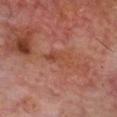patient: male, aged 63–67 | automated lesion analysis: a classifier nevus-likeness of about 0/100 and a detector confidence of about 100 out of 100 that the crop contains a lesion | imaging modality: ~15 mm tile from a whole-body skin photo | body site: the head or neck | diameter: ~3 mm (longest diameter).